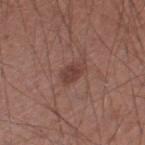follow-up = total-body-photography surveillance lesion; no biopsy | image = ~15 mm crop, total-body skin-cancer survey | diameter = ≈3 mm | subject = male, in their mid-50s | illumination = white-light | anatomic site = the leg.